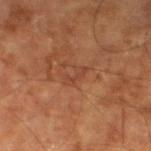lighting: cross-polarized | lesion size: about 3 mm | subject: male, approximately 65 years of age | anatomic site: the left lower leg | acquisition: ~15 mm tile from a whole-body skin photo | automated lesion analysis: a nevus-likeness score of about 0/100 and a lesion-detection confidence of about 95/100.